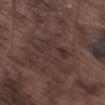Imaged during a routine full-body skin examination; the lesion was not biopsied and no histopathology is available. The tile uses white-light illumination. The patient is a male roughly 75 years of age. A close-up tile cropped from a whole-body skin photograph, about 15 mm across. About 7.5 mm across. Located on the left thigh.